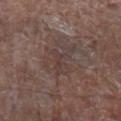Recorded during total-body skin imaging; not selected for excision or biopsy. This is a white-light tile. A close-up tile cropped from a whole-body skin photograph, about 15 mm across. The lesion is located on the left forearm. About 5.5 mm across. A male patient in their 80s.